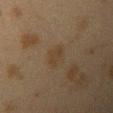{
  "biopsy_status": "not biopsied; imaged during a skin examination",
  "image": {
    "source": "total-body photography crop",
    "field_of_view_mm": 15
  },
  "lighting": "cross-polarized",
  "site": "arm",
  "patient": {
    "sex": "female",
    "age_approx": 40
  }
}